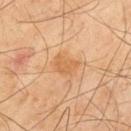Clinical impression:
Imaged during a routine full-body skin examination; the lesion was not biopsied and no histopathology is available.
Background:
On the mid back. A male subject, aged approximately 45. Cropped from a total-body skin-imaging series; the visible field is about 15 mm.